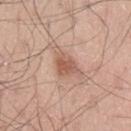Imaged during a routine full-body skin examination; the lesion was not biopsied and no histopathology is available. A lesion tile, about 15 mm wide, cut from a 3D total-body photograph. The patient is a male aged approximately 70. Automated image analysis of the tile measured roughly 10 lightness units darker than nearby skin and a normalized lesion–skin contrast near 7. The analysis additionally found an automated nevus-likeness rating near 70 out of 100. Captured under white-light illumination. Located on the lower back. Measured at roughly 2.5 mm in maximum diameter.